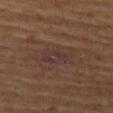  biopsy_status: not biopsied; imaged during a skin examination
  image:
    source: total-body photography crop
    field_of_view_mm: 15
  lighting: cross-polarized
  lesion_size:
    long_diameter_mm_approx: 4.0
  site: left thigh
  patient:
    sex: male
    age_approx: 65
  automated_metrics:
    area_mm2_approx: 6.5
    cielab_L: 29
    cielab_a: 15
    cielab_b: 17
    vs_skin_darker_L: 4.0
    nevus_likeness_0_100: 0